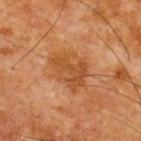Impression: No biopsy was performed on this lesion — it was imaged during a full skin examination and was not determined to be concerning.